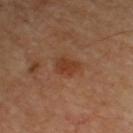Recorded during total-body skin imaging; not selected for excision or biopsy. Captured under cross-polarized illumination. Cropped from a total-body skin-imaging series; the visible field is about 15 mm. About 3 mm across. On the right upper arm.